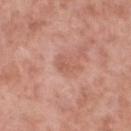<record>
  <biopsy_status>not biopsied; imaged during a skin examination</biopsy_status>
  <image>
    <source>total-body photography crop</source>
    <field_of_view_mm>15</field_of_view_mm>
  </image>
  <lighting>white-light</lighting>
  <site>left lower leg</site>
  <automated_metrics>
    <nevus_likeness_0_100>0</nevus_likeness_0_100>
    <lesion_detection_confidence_0_100>100</lesion_detection_confidence_0_100>
  </automated_metrics>
  <lesion_size>
    <long_diameter_mm_approx>3.0</long_diameter_mm_approx>
  </lesion_size>
  <patient>
    <sex>male</sex>
    <age_approx>55</age_approx>
  </patient>
</record>